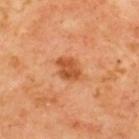Recorded during total-body skin imaging; not selected for excision or biopsy. This is a cross-polarized tile. Approximately 3 mm at its widest. The patient is aged around 65. A 15 mm close-up extracted from a 3D total-body photography capture. Automated tile analysis of the lesion measured a lesion area of about 6 mm², an outline eccentricity of about 0.7 (0 = round, 1 = elongated), and a shape-asymmetry score of about 0.15 (0 = symmetric). The software also gave a border-irregularity rating of about 1.5/10 and peripheral color asymmetry of about 1. The lesion is on the upper back.